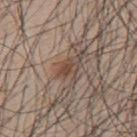Part of a total-body skin-imaging series; this lesion was reviewed on a skin check and was not flagged for biopsy.
The tile uses white-light illumination.
Automated tile analysis of the lesion measured an average lesion color of about L≈45 a*≈16 b*≈25 (CIELAB), a lesion–skin lightness drop of about 9, and a lesion-to-skin contrast of about 7 (normalized; higher = more distinct). And it measured border irregularity of about 3 on a 0–10 scale, a within-lesion color-variation index near 2.5/10, and peripheral color asymmetry of about 1. The analysis additionally found an automated nevus-likeness rating near 85 out of 100.
The lesion is on the upper back.
The subject is a male aged around 45.
Cropped from a total-body skin-imaging series; the visible field is about 15 mm.
The recorded lesion diameter is about 2.5 mm.